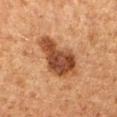{"image": {"source": "total-body photography crop", "field_of_view_mm": 15}, "patient": {"sex": "female", "age_approx": 40}, "lesion_size": {"long_diameter_mm_approx": 6.0}, "lighting": "cross-polarized", "site": "right forearm"}